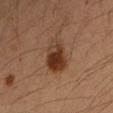  biopsy_status: not biopsied; imaged during a skin examination
  patient:
    sex: female
    age_approx: 35
  image:
    source: total-body photography crop
    field_of_view_mm: 15
  site: left forearm